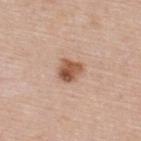biopsy status: total-body-photography surveillance lesion; no biopsy | tile lighting: white-light | subject: female, aged 48 to 52 | size: ≈3 mm | location: the upper back | imaging modality: ~15 mm crop, total-body skin-cancer survey.